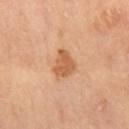biopsy status = total-body-photography surveillance lesion; no biopsy
diameter = ~3.5 mm (longest diameter)
automated metrics = an area of roughly 6.5 mm², an eccentricity of roughly 0.6, and a symmetry-axis asymmetry near 0.35; a lesion–skin lightness drop of about 11
body site = the left leg
image = ~15 mm crop, total-body skin-cancer survey
lighting = cross-polarized
patient = female, aged around 60